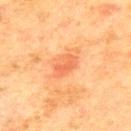notes: catalogued during a skin exam; not biopsied | image source: ~15 mm tile from a whole-body skin photo | tile lighting: cross-polarized illumination | image-analysis metrics: a lesion area of about 4 mm², a shape eccentricity near 0.85, and two-axis asymmetry of about 0.35; a mean CIELAB color near L≈55 a*≈29 b*≈38 and a lesion-to-skin contrast of about 5.5 (normalized; higher = more distinct); a border-irregularity rating of about 4/10 and internal color variation of about 3.5 on a 0–10 scale | subject: male, approximately 70 years of age | anatomic site: the upper back.